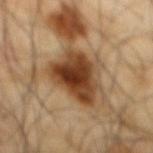This lesion was catalogued during total-body skin photography and was not selected for biopsy.
A close-up tile cropped from a whole-body skin photograph, about 15 mm across.
From the mid back.
A male patient, aged 63 to 67.